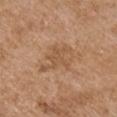Q: Was this lesion biopsied?
A: total-body-photography surveillance lesion; no biopsy
Q: What is the anatomic site?
A: the arm
Q: How was the tile lit?
A: white-light
Q: Patient demographics?
A: male, about 75 years old
Q: Automated lesion metrics?
A: a classifier nevus-likeness of about 0/100 and a lesion-detection confidence of about 100/100
Q: How was this image acquired?
A: total-body-photography crop, ~15 mm field of view